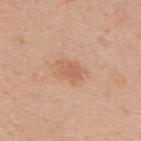| feature | finding |
|---|---|
| notes | total-body-photography surveillance lesion; no biopsy |
| automated lesion analysis | a footprint of about 4.5 mm², a shape eccentricity near 0.75, and a symmetry-axis asymmetry near 0.2 |
| acquisition | total-body-photography crop, ~15 mm field of view |
| subject | female, aged approximately 30 |
| site | the upper back |
| lesion size | ≈3 mm |
| lighting | white-light |